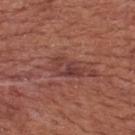No biopsy was performed on this lesion — it was imaged during a full skin examination and was not determined to be concerning. About 4 mm across. The lesion is on the back. This image is a 15 mm lesion crop taken from a total-body photograph. A male patient aged 63–67. Captured under white-light illumination.